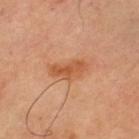Findings:
- biopsy status · total-body-photography surveillance lesion; no biopsy
- acquisition · 15 mm crop, total-body photography
- lesion size · ≈4.5 mm
- tile lighting · cross-polarized illumination
- image-analysis metrics · a footprint of about 7 mm² and a shape eccentricity near 0.9; an average lesion color of about L≈56 a*≈26 b*≈39 (CIELAB), about 9 CIELAB-L* units darker than the surrounding skin, and a lesion-to-skin contrast of about 7 (normalized; higher = more distinct); a border-irregularity rating of about 4/10 and a within-lesion color-variation index near 3/10; a nevus-likeness score of about 30/100 and a lesion-detection confidence of about 100/100
- subject · male, aged 63 to 67
- location · the left thigh